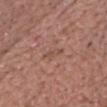Acquisition and patient details:
The tile uses white-light illumination. The lesion is on the chest. Approximately 3 mm at its widest. A lesion tile, about 15 mm wide, cut from a 3D total-body photograph. The total-body-photography lesion software estimated a footprint of about 2.5 mm² and a shape eccentricity near 0.95. The software also gave a lesion color around L≈49 a*≈20 b*≈27 in CIELAB, a lesion–skin lightness drop of about 6, and a normalized lesion–skin contrast near 5. The analysis additionally found a border-irregularity index near 4/10 and a color-variation rating of about 0/10. A male subject aged 53 to 57.